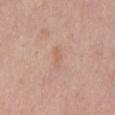Imaged during a routine full-body skin examination; the lesion was not biopsied and no histopathology is available.
A lesion tile, about 15 mm wide, cut from a 3D total-body photograph.
The patient is a male in their 40s.
The lesion is located on the mid back.